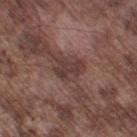Recorded during total-body skin imaging; not selected for excision or biopsy. A male patient in their mid- to late 70s. The total-body-photography lesion software estimated a border-irregularity rating of about 5.5/10, a within-lesion color-variation index near 0/10, and a peripheral color-asymmetry measure near 0. A lesion tile, about 15 mm wide, cut from a 3D total-body photograph. Longest diameter approximately 3 mm. From the left thigh.